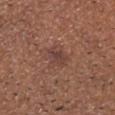notes = catalogued during a skin exam; not biopsied
lighting = white-light illumination
acquisition = ~15 mm crop, total-body skin-cancer survey
lesion size = ~3 mm (longest diameter)
location = the head or neck
subject = male, aged 63–67